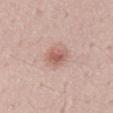workup — imaged on a skin check; not biopsied
acquisition — total-body-photography crop, ~15 mm field of view
patient — male, about 35 years old
anatomic site — the back
automated lesion analysis — an eccentricity of roughly 0.4 and a shape-asymmetry score of about 0.3 (0 = symmetric); a mean CIELAB color near L≈59 a*≈21 b*≈26 and about 10 CIELAB-L* units darker than the surrounding skin